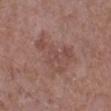Recorded during total-body skin imaging; not selected for excision or biopsy. On the leg. A male subject, roughly 70 years of age. A region of skin cropped from a whole-body photographic capture, roughly 15 mm wide.